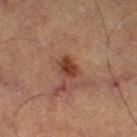<case>
  <biopsy_status>not biopsied; imaged during a skin examination</biopsy_status>
  <lighting>cross-polarized</lighting>
  <lesion_size>
    <long_diameter_mm_approx>2.5</long_diameter_mm_approx>
  </lesion_size>
  <site>right thigh</site>
  <patient>
    <sex>female</sex>
    <age_approx>60</age_approx>
  </patient>
  <image>
    <source>total-body photography crop</source>
    <field_of_view_mm>15</field_of_view_mm>
  </image>
</case>